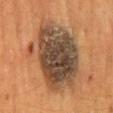This lesion was catalogued during total-body skin photography and was not selected for biopsy. A male patient aged 53 to 57. The lesion is on the mid back. The tile uses cross-polarized illumination. The lesion's longest dimension is about 10 mm. This image is a 15 mm lesion crop taken from a total-body photograph.